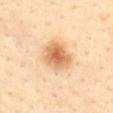Context: The lesion is located on the mid back. A female patient, aged approximately 40. Captured under cross-polarized illumination. This image is a 15 mm lesion crop taken from a total-body photograph.